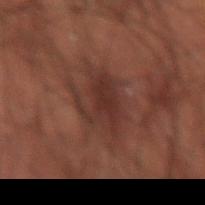Clinical impression:
This lesion was catalogued during total-body skin photography and was not selected for biopsy.
Context:
Automated image analysis of the tile measured a lesion area of about 13 mm², an outline eccentricity of about 0.6 (0 = round, 1 = elongated), and a symmetry-axis asymmetry near 0.4. And it measured border irregularity of about 7 on a 0–10 scale, a within-lesion color-variation index near 2/10, and radial color variation of about 0.5. The lesion's longest dimension is about 5.5 mm. Captured under cross-polarized illumination. From the back. A male patient, roughly 50 years of age. A roughly 15 mm field-of-view crop from a total-body skin photograph.